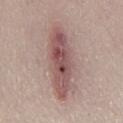Assessment: Recorded during total-body skin imaging; not selected for excision or biopsy. Background: Approximately 9 mm at its widest. The tile uses white-light illumination. A lesion tile, about 15 mm wide, cut from a 3D total-body photograph. From the left lower leg. A female patient aged around 60.